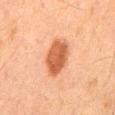workup = catalogued during a skin exam; not biopsied.